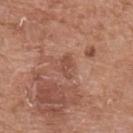| key | value |
|---|---|
| subject | male, aged approximately 80 |
| imaging modality | total-body-photography crop, ~15 mm field of view |
| size | ≈2.5 mm |
| body site | the arm |
| image-analysis metrics | a lesion color around L≈49 a*≈23 b*≈28 in CIELAB and a normalized lesion–skin contrast near 6; a border-irregularity index near 4/10, a within-lesion color-variation index near 1/10, and peripheral color asymmetry of about 0.5; a classifier nevus-likeness of about 0/100 and a detector confidence of about 100 out of 100 that the crop contains a lesion |
| lighting | white-light illumination |